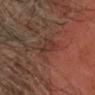- workup — no biopsy performed (imaged during a skin exam)
- body site — the head or neck
- image — 15 mm crop, total-body photography
- subject — male, about 60 years old
- lighting — cross-polarized illumination
- automated metrics — a lesion area of about 6 mm² and two-axis asymmetry of about 0.3; roughly 5 lightness units darker than nearby skin
- lesion size — ~3.5 mm (longest diameter)